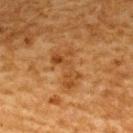biopsy status: total-body-photography surveillance lesion; no biopsy | body site: the upper back | imaging modality: 15 mm crop, total-body photography | lighting: cross-polarized illumination | subject: male, aged 58 to 62.